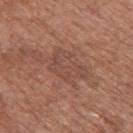The patient is a male in their mid-70s. This image is a 15 mm lesion crop taken from a total-body photograph. The lesion is located on the upper back.